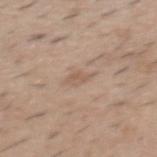biopsy status = catalogued during a skin exam; not biopsied | automated lesion analysis = about 7 CIELAB-L* units darker than the surrounding skin and a normalized lesion–skin contrast near 5; a border-irregularity rating of about 2.5/10 and peripheral color asymmetry of about 0.5 | lesion size = about 2.5 mm | subject = male, aged 38–42 | tile lighting = white-light | body site = the mid back | image = ~15 mm crop, total-body skin-cancer survey.